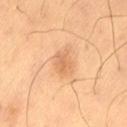The lesion's longest dimension is about 2.5 mm. A 15 mm close-up tile from a total-body photography series done for melanoma screening. This is a cross-polarized tile. Automated tile analysis of the lesion measured border irregularity of about 2.5 on a 0–10 scale and a within-lesion color-variation index near 2.5/10. The software also gave a classifier nevus-likeness of about 5/100 and lesion-presence confidence of about 100/100. Located on the lower back. A male patient, in their 60s.